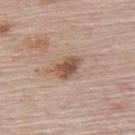Notes:
– workup — total-body-photography surveillance lesion; no biopsy
– subject — female, aged around 65
– image source — ~15 mm tile from a whole-body skin photo
– location — the upper back
– automated lesion analysis — a footprint of about 6 mm², an outline eccentricity of about 0.75 (0 = round, 1 = elongated), and a shape-asymmetry score of about 0.2 (0 = symmetric); about 12 CIELAB-L* units darker than the surrounding skin and a normalized lesion–skin contrast near 8.5; a border-irregularity rating of about 2/10, internal color variation of about 4 on a 0–10 scale, and radial color variation of about 1
– lesion diameter — ~3.5 mm (longest diameter)